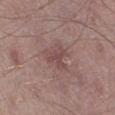Q: What is the anatomic site?
A: the right lower leg
Q: Automated lesion metrics?
A: an outline eccentricity of about 0.65 (0 = round, 1 = elongated); about 7 CIELAB-L* units darker than the surrounding skin and a lesion-to-skin contrast of about 5.5 (normalized; higher = more distinct); a peripheral color-asymmetry measure near 0
Q: Lesion size?
A: about 3 mm
Q: What is the imaging modality?
A: ~15 mm tile from a whole-body skin photo
Q: Patient demographics?
A: male, aged 63–67
Q: Illumination type?
A: white-light